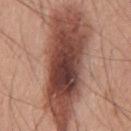Recorded during total-body skin imaging; not selected for excision or biopsy.
On the abdomen.
The subject is a male roughly 60 years of age.
Automated tile analysis of the lesion measured an area of roughly 60 mm² and two-axis asymmetry of about 0.25. The software also gave a lesion color around L≈46 a*≈22 b*≈26 in CIELAB, a lesion–skin lightness drop of about 17, and a normalized border contrast of about 11.5. And it measured a border-irregularity index near 4.5/10 and peripheral color asymmetry of about 2. It also reported an automated nevus-likeness rating near 35 out of 100 and lesion-presence confidence of about 100/100.
Cropped from a total-body skin-imaging series; the visible field is about 15 mm.
The lesion's longest dimension is about 15.5 mm.
Captured under white-light illumination.